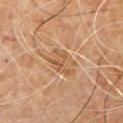The lesion was tiled from a total-body skin photograph and was not biopsied.